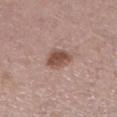Imaged during a routine full-body skin examination; the lesion was not biopsied and no histopathology is available.